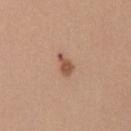This lesion was catalogued during total-body skin photography and was not selected for biopsy. A male patient in their 40s. The lesion is on the chest. A region of skin cropped from a whole-body photographic capture, roughly 15 mm wide.